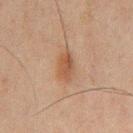biopsy status: no biopsy performed (imaged during a skin exam) | patient: male, aged approximately 45 | lesion size: about 3.5 mm | acquisition: total-body-photography crop, ~15 mm field of view | image-analysis metrics: a border-irregularity rating of about 2.5/10 and radial color variation of about 1; a nevus-likeness score of about 35/100 and a detector confidence of about 100 out of 100 that the crop contains a lesion | site: the chest | illumination: cross-polarized.